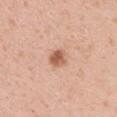Impression: Imaged during a routine full-body skin examination; the lesion was not biopsied and no histopathology is available. Image and clinical context: Located on the left upper arm. A male subject approximately 30 years of age. Cropped from a total-body skin-imaging series; the visible field is about 15 mm.